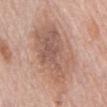<case>
  <site>chest</site>
  <patient>
    <sex>female</sex>
    <age_approx>65</age_approx>
  </patient>
  <automated_metrics>
    <shape_asymmetry>0.2</shape_asymmetry>
    <border_irregularity_0_10>3.5</border_irregularity_0_10>
    <color_variation_0_10>5.5</color_variation_0_10>
    <peripheral_color_asymmetry>2.0</peripheral_color_asymmetry>
    <nevus_likeness_0_100>0</nevus_likeness_0_100>
    <lesion_detection_confidence_0_100>100</lesion_detection_confidence_0_100>
  </automated_metrics>
  <lesion_size>
    <long_diameter_mm_approx>10.0</long_diameter_mm_approx>
  </lesion_size>
  <lighting>white-light</lighting>
  <image>
    <source>total-body photography crop</source>
    <field_of_view_mm>15</field_of_view_mm>
  </image>
</case>